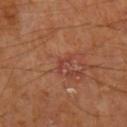tile lighting — cross-polarized illumination; lesion diameter — about 3 mm; location — the arm; image source — 15 mm crop, total-body photography.